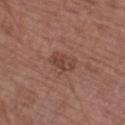notes: imaged on a skin check; not biopsied | TBP lesion metrics: an area of roughly 6 mm², an eccentricity of roughly 0.75, and a shape-asymmetry score of about 0.2 (0 = symmetric); a lesion–skin lightness drop of about 7 and a lesion-to-skin contrast of about 6 (normalized; higher = more distinct); a border-irregularity index near 2.5/10, internal color variation of about 3.5 on a 0–10 scale, and peripheral color asymmetry of about 1.5; a lesion-detection confidence of about 100/100 | body site: the left forearm | imaging modality: total-body-photography crop, ~15 mm field of view | patient: male, about 75 years old | tile lighting: white-light.